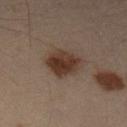No biopsy was performed on this lesion — it was imaged during a full skin examination and was not determined to be concerning. The lesion's longest dimension is about 4.5 mm. The subject is a male in their mid-50s. Captured under cross-polarized illumination. A region of skin cropped from a whole-body photographic capture, roughly 15 mm wide. The lesion is on the left lower leg.